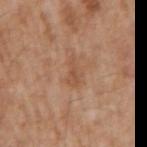{"biopsy_status": "not biopsied; imaged during a skin examination", "lesion_size": {"long_diameter_mm_approx": 3.0}, "image": {"source": "total-body photography crop", "field_of_view_mm": 15}, "patient": {"sex": "male", "age_approx": 65}, "automated_metrics": {"cielab_L": 52, "cielab_a": 21, "cielab_b": 33, "vs_skin_darker_L": 7.0, "vs_skin_contrast_norm": 5.5, "border_irregularity_0_10": 6.5, "color_variation_0_10": 0.5, "nevus_likeness_0_100": 0, "lesion_detection_confidence_0_100": 100}, "site": "arm"}